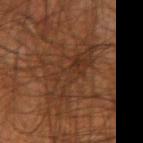No biopsy was performed on this lesion — it was imaged during a full skin examination and was not determined to be concerning.
The lesion is located on the left forearm.
Measured at roughly 7.5 mm in maximum diameter.
A male patient, approximately 45 years of age.
Automated image analysis of the tile measured an eccentricity of roughly 0.9 and a symmetry-axis asymmetry near 0.45. And it measured a mean CIELAB color near L≈31 a*≈19 b*≈28 and about 5 CIELAB-L* units darker than the surrounding skin. It also reported a border-irregularity rating of about 8.5/10. The software also gave lesion-presence confidence of about 55/100.
A 15 mm crop from a total-body photograph taken for skin-cancer surveillance.